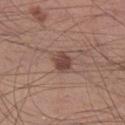Q: Was a biopsy performed?
A: no biopsy performed (imaged during a skin exam)
Q: What are the patient's age and sex?
A: male, aged around 30
Q: What kind of image is this?
A: 15 mm crop, total-body photography
Q: Lesion location?
A: the right lower leg